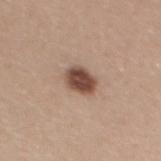No biopsy was performed on this lesion — it was imaged during a full skin examination and was not determined to be concerning. The patient is a female aged 28 to 32. This is a white-light tile. The lesion's longest dimension is about 3 mm. A close-up tile cropped from a whole-body skin photograph, about 15 mm across. On the upper back.